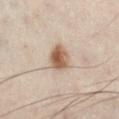Notes:
– workup · no biopsy performed (imaged during a skin exam)
– subject · male, roughly 50 years of age
– image source · ~15 mm tile from a whole-body skin photo
– anatomic site · the leg
– automated lesion analysis · a lesion color around L≈48 a*≈13 b*≈24 in CIELAB, a lesion–skin lightness drop of about 11, and a lesion-to-skin contrast of about 8.5 (normalized; higher = more distinct); a border-irregularity rating of about 2/10, a color-variation rating of about 6/10, and peripheral color asymmetry of about 2; an automated nevus-likeness rating near 100 out of 100
– illumination · cross-polarized